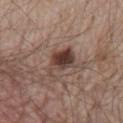Captured during whole-body skin photography for melanoma surveillance; the lesion was not biopsied.
Located on the front of the torso.
A 15 mm crop from a total-body photograph taken for skin-cancer surveillance.
A male subject, aged 78–82.